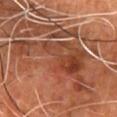biopsy status: imaged on a skin check; not biopsied
subject: male, aged approximately 60
imaging modality: 15 mm crop, total-body photography
image-analysis metrics: an area of roughly 80 mm² and a shape-asymmetry score of about 0.35 (0 = symmetric); an average lesion color of about L≈42 a*≈23 b*≈29 (CIELAB), roughly 8 lightness units darker than nearby skin, and a normalized lesion–skin contrast near 6.5; a border-irregularity index near 6.5/10 and a color-variation rating of about 8.5/10; a nevus-likeness score of about 0/100 and a detector confidence of about 85 out of 100 that the crop contains a lesion
site: the chest
diameter: about 13.5 mm
illumination: cross-polarized illumination